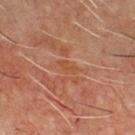Findings:
• biopsy status — no biopsy performed (imaged during a skin exam)
• lesion size — about 2.5 mm
• patient — male, aged around 60
• illumination — cross-polarized illumination
• automated lesion analysis — border irregularity of about 4 on a 0–10 scale; an automated nevus-likeness rating near 0 out of 100 and a detector confidence of about 60 out of 100 that the crop contains a lesion
• location — the chest
• acquisition — ~15 mm tile from a whole-body skin photo